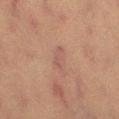Recorded during total-body skin imaging; not selected for excision or biopsy. The lesion is located on the left thigh. Automated tile analysis of the lesion measured a footprint of about 4.5 mm² and two-axis asymmetry of about 0.35. The software also gave a lesion color around L≈43 a*≈18 b*≈21 in CIELAB and a lesion-to-skin contrast of about 4.5 (normalized; higher = more distinct). A 15 mm close-up tile from a total-body photography series done for melanoma screening. About 3.5 mm across. The patient is a female aged approximately 55. Captured under cross-polarized illumination.